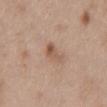Clinical impression:
The lesion was photographed on a routine skin check and not biopsied; there is no pathology result.